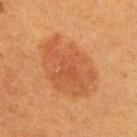No biopsy was performed on this lesion — it was imaged during a full skin examination and was not determined to be concerning.